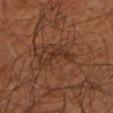Notes:
– follow-up: no biopsy performed (imaged during a skin exam)
– size: ~4.5 mm (longest diameter)
– automated lesion analysis: a nevus-likeness score of about 0/100
– image source: 15 mm crop, total-body photography
– lighting: cross-polarized
– patient: in their mid- to late 60s
– anatomic site: the left lower leg